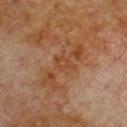Imaged during a routine full-body skin examination; the lesion was not biopsied and no histopathology is available. A region of skin cropped from a whole-body photographic capture, roughly 15 mm wide. The subject is a male aged 78 to 82. The lesion is on the chest.